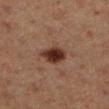Imaged during a routine full-body skin examination; the lesion was not biopsied and no histopathology is available. The recorded lesion diameter is about 3.5 mm. A female patient, approximately 40 years of age. An algorithmic analysis of the crop reported a mean CIELAB color near L≈26 a*≈18 b*≈22, about 12 CIELAB-L* units darker than the surrounding skin, and a lesion-to-skin contrast of about 12 (normalized; higher = more distinct). It also reported a nevus-likeness score of about 100/100 and a lesion-detection confidence of about 100/100. A roughly 15 mm field-of-view crop from a total-body skin photograph. From the left lower leg. Captured under cross-polarized illumination.